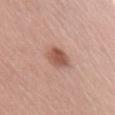Imaged during a routine full-body skin examination; the lesion was not biopsied and no histopathology is available. Imaged with white-light lighting. Longest diameter approximately 3 mm. A 15 mm crop from a total-body photograph taken for skin-cancer surveillance. Automated tile analysis of the lesion measured a mean CIELAB color near L≈54 a*≈23 b*≈28, a lesion–skin lightness drop of about 12, and a lesion-to-skin contrast of about 8 (normalized; higher = more distinct). Located on the right upper arm. A female subject in their 40s.